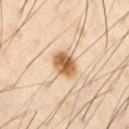No biopsy was performed on this lesion — it was imaged during a full skin examination and was not determined to be concerning. A 15 mm close-up tile from a total-body photography series done for melanoma screening. A male subject aged 53 to 57. From the right thigh.